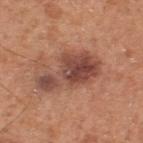Assessment:
Captured during whole-body skin photography for melanoma surveillance; the lesion was not biopsied.
Image and clinical context:
Automated tile analysis of the lesion measured a lesion area of about 19 mm², a shape eccentricity near 0.85, and two-axis asymmetry of about 0.35. About 7 mm across. On the upper back. A region of skin cropped from a whole-body photographic capture, roughly 15 mm wide. A male patient aged around 55.